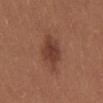| feature | finding |
|---|---|
| patient | female, in their mid- to late 20s |
| site | the mid back |
| imaging modality | ~15 mm crop, total-body skin-cancer survey |
| tile lighting | white-light illumination |
| lesion size | ≈5 mm |
| TBP lesion metrics | a mean CIELAB color near L≈40 a*≈23 b*≈27, roughly 10 lightness units darker than nearby skin, and a normalized lesion–skin contrast near 8; internal color variation of about 3 on a 0–10 scale and a peripheral color-asymmetry measure near 1 |